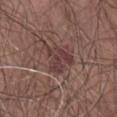<tbp_lesion>
  <biopsy_status>not biopsied; imaged during a skin examination</biopsy_status>
  <site>abdomen</site>
  <image>
    <source>total-body photography crop</source>
    <field_of_view_mm>15</field_of_view_mm>
  </image>
  <patient>
    <sex>male</sex>
    <age_approx>75</age_approx>
  </patient>
  <lighting>white-light</lighting>
  <automated_metrics>
    <color_variation_0_10>4.5</color_variation_0_10>
    <peripheral_color_asymmetry>1.5</peripheral_color_asymmetry>
    <lesion_detection_confidence_0_100>90</lesion_detection_confidence_0_100>
  </automated_metrics>
</tbp_lesion>